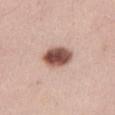biopsy_status: not biopsied; imaged during a skin examination
lighting: white-light
automated_metrics:
  border_irregularity_0_10: 2.0
  color_variation_0_10: 6.0
  lesion_detection_confidence_0_100: 100
patient:
  sex: female
  age_approx: 35
lesion_size:
  long_diameter_mm_approx: 4.0
site: right thigh
image:
  source: total-body photography crop
  field_of_view_mm: 15